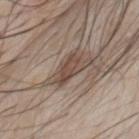<case>
  <biopsy_status>not biopsied; imaged during a skin examination</biopsy_status>
  <lesion_size>
    <long_diameter_mm_approx>4.0</long_diameter_mm_approx>
  </lesion_size>
  <lighting>white-light</lighting>
  <patient>
    <sex>male</sex>
    <age_approx>60</age_approx>
  </patient>
  <image>
    <source>total-body photography crop</source>
    <field_of_view_mm>15</field_of_view_mm>
  </image>
  <site>chest</site>
  <automated_metrics>
    <nevus_likeness_0_100>60</nevus_likeness_0_100>
    <lesion_detection_confidence_0_100>90</lesion_detection_confidence_0_100>
  </automated_metrics>
</case>